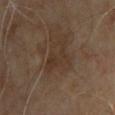workup: no biopsy performed (imaged during a skin exam)
image: ~15 mm crop, total-body skin-cancer survey
patient: male, aged 63–67
site: the left upper arm
tile lighting: cross-polarized
lesion size: about 4.5 mm